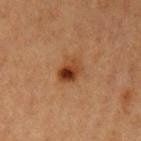follow-up=no biopsy performed (imaged during a skin exam).